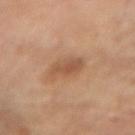Assessment:
The lesion was tiled from a total-body skin photograph and was not biopsied.
Context:
A male subject roughly 55 years of age. From the right forearm. The recorded lesion diameter is about 3.5 mm. The tile uses cross-polarized illumination. A 15 mm close-up tile from a total-body photography series done for melanoma screening.